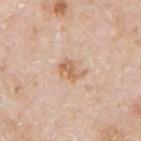Clinical impression: No biopsy was performed on this lesion — it was imaged during a full skin examination and was not determined to be concerning. Context: A region of skin cropped from a whole-body photographic capture, roughly 15 mm wide. The patient is a male aged 73–77. Imaged with white-light lighting. On the arm.